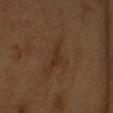Part of a total-body skin-imaging series; this lesion was reviewed on a skin check and was not flagged for biopsy.
The lesion-visualizer software estimated a footprint of about 2.5 mm², an outline eccentricity of about 0.9 (0 = round, 1 = elongated), and two-axis asymmetry of about 0.4. The software also gave lesion-presence confidence of about 95/100.
On the right forearm.
Cropped from a whole-body photographic skin survey; the tile spans about 15 mm.
A female patient, roughly 55 years of age.
Longest diameter approximately 2.5 mm.